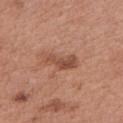Imaged during a routine full-body skin examination; the lesion was not biopsied and no histopathology is available.
Captured under white-light illumination.
A 15 mm close-up tile from a total-body photography series done for melanoma screening.
The subject is a female roughly 60 years of age.
From the right upper arm.